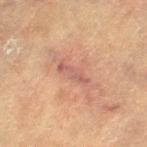Impression:
Recorded during total-body skin imaging; not selected for excision or biopsy.
Acquisition and patient details:
Automated tile analysis of the lesion measured a lesion area of about 4 mm², an outline eccentricity of about 0.95 (0 = round, 1 = elongated), and a symmetry-axis asymmetry near 0.3. And it measured a normalized border contrast of about 6. The lesion is on the left thigh. The subject is a female aged around 80. This image is a 15 mm lesion crop taken from a total-body photograph.